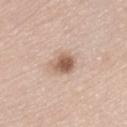Q: Was a biopsy performed?
A: total-body-photography surveillance lesion; no biopsy
Q: Automated lesion metrics?
A: a lesion area of about 6.5 mm², a shape eccentricity near 0.65, and two-axis asymmetry of about 0.25; a mean CIELAB color near L≈59 a*≈18 b*≈28, about 14 CIELAB-L* units darker than the surrounding skin, and a lesion-to-skin contrast of about 8.5 (normalized; higher = more distinct); border irregularity of about 2.5 on a 0–10 scale and peripheral color asymmetry of about 2
Q: Illumination type?
A: white-light
Q: Lesion location?
A: the left thigh
Q: Who is the patient?
A: female, aged around 75
Q: What is the imaging modality?
A: total-body-photography crop, ~15 mm field of view
Q: What is the lesion's diameter?
A: ≈3.5 mm